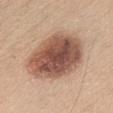Q: Was this lesion biopsied?
A: no biopsy performed (imaged during a skin exam)
Q: What are the patient's age and sex?
A: female, about 40 years old
Q: Lesion location?
A: the chest
Q: Automated lesion metrics?
A: a shape eccentricity near 0.75 and two-axis asymmetry of about 0.1; an average lesion color of about L≈53 a*≈20 b*≈28 (CIELAB), about 17 CIELAB-L* units darker than the surrounding skin, and a normalized border contrast of about 11
Q: Illumination type?
A: white-light
Q: Lesion size?
A: about 8 mm
Q: What is the imaging modality?
A: total-body-photography crop, ~15 mm field of view